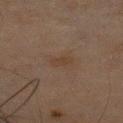notes: total-body-photography surveillance lesion; no biopsy | body site: the left upper arm | patient: male, roughly 65 years of age | image: ~15 mm crop, total-body skin-cancer survey.